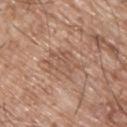biopsy status: no biopsy performed (imaged during a skin exam) | automated metrics: a lesion color around L≈55 a*≈19 b*≈29 in CIELAB, roughly 7 lightness units darker than nearby skin, and a normalized border contrast of about 5; lesion-presence confidence of about 95/100 | site: the upper back | acquisition: ~15 mm crop, total-body skin-cancer survey | lesion size: ~5 mm (longest diameter) | patient: male, in their mid- to late 60s | illumination: white-light.